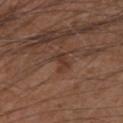The lesion was photographed on a routine skin check and not biopsied; there is no pathology result. The lesion is located on the arm. A close-up tile cropped from a whole-body skin photograph, about 15 mm across. A male patient, aged around 50.